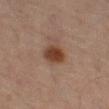No biopsy was performed on this lesion — it was imaged during a full skin examination and was not determined to be concerning. Longest diameter approximately 3 mm. This is a cross-polarized tile. On the right thigh. The patient is a male aged approximately 70. A roughly 15 mm field-of-view crop from a total-body skin photograph.